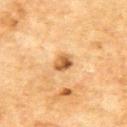Captured during whole-body skin photography for melanoma surveillance; the lesion was not biopsied. A 15 mm crop from a total-body photograph taken for skin-cancer surveillance. A male subject, aged 68–72. Automated image analysis of the tile measured a nevus-likeness score of about 90/100 and lesion-presence confidence of about 100/100. This is a cross-polarized tile. From the upper back.